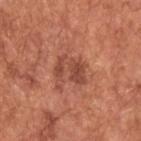  lesion_size:
    long_diameter_mm_approx: 4.0
  patient:
    sex: male
    age_approx: 65
  lighting: white-light
  automated_metrics:
    area_mm2_approx: 8.0
    eccentricity: 0.75
    shape_asymmetry: 0.35
    border_irregularity_0_10: 4.5
    color_variation_0_10: 5.0
    peripheral_color_asymmetry: 2.0
    nevus_likeness_0_100: 30
  site: upper back
  image:
    source: total-body photography crop
    field_of_view_mm: 15